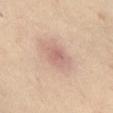Imaged during a routine full-body skin examination; the lesion was not biopsied and no histopathology is available.
A region of skin cropped from a whole-body photographic capture, roughly 15 mm wide.
About 3.5 mm across.
The total-body-photography lesion software estimated a shape eccentricity near 0.7. The analysis additionally found a lesion color around L≈64 a*≈20 b*≈26 in CIELAB and about 8 CIELAB-L* units darker than the surrounding skin.
A female subject aged 53 to 57.
Captured under cross-polarized illumination.
The lesion is on the abdomen.